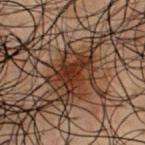Assessment:
Imaged during a routine full-body skin examination; the lesion was not biopsied and no histopathology is available.
Background:
The patient is a male aged 48 to 52. Captured under cross-polarized illumination. Longest diameter approximately 4.5 mm. Cropped from a whole-body photographic skin survey; the tile spans about 15 mm. Located on the chest. An algorithmic analysis of the crop reported a lesion area of about 8.5 mm², a shape eccentricity near 0.8, and two-axis asymmetry of about 0.5. The software also gave a border-irregularity rating of about 5.5/10 and radial color variation of about 1. And it measured a detector confidence of about 55 out of 100 that the crop contains a lesion.